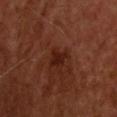Assessment:
The lesion was tiled from a total-body skin photograph and was not biopsied.
Acquisition and patient details:
This is a cross-polarized tile. Located on the back. A 15 mm close-up tile from a total-body photography series done for melanoma screening. A male subject, in their mid-50s.